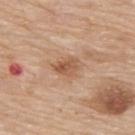Part of a total-body skin-imaging series; this lesion was reviewed on a skin check and was not flagged for biopsy. Cropped from a whole-body photographic skin survey; the tile spans about 15 mm. The subject is a male aged 78–82. Automated tile analysis of the lesion measured an area of roughly 6 mm², an eccentricity of roughly 0.75, and a symmetry-axis asymmetry near 0.25. It also reported a border-irregularity index near 2.5/10 and internal color variation of about 5 on a 0–10 scale. From the upper back. This is a white-light tile. About 3.5 mm across.